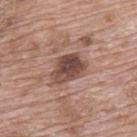The lesion was photographed on a routine skin check and not biopsied; there is no pathology result. The lesion is located on the upper back. The lesion's longest dimension is about 4.5 mm. The tile uses white-light illumination. The total-body-photography lesion software estimated a detector confidence of about 100 out of 100 that the crop contains a lesion. A male subject in their 70s. A 15 mm close-up tile from a total-body photography series done for melanoma screening.